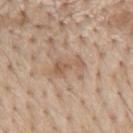<lesion>
  <biopsy_status>not biopsied; imaged during a skin examination</biopsy_status>
  <automated_metrics>
    <cielab_L>57</cielab_L>
    <cielab_a>17</cielab_a>
    <cielab_b>30</cielab_b>
    <vs_skin_darker_L>8.0</vs_skin_darker_L>
    <vs_skin_contrast_norm>5.5</vs_skin_contrast_norm>
    <border_irregularity_0_10>6.5</border_irregularity_0_10>
    <color_variation_0_10>2.0</color_variation_0_10>
    <peripheral_color_asymmetry>0.5</peripheral_color_asymmetry>
    <lesion_detection_confidence_0_100>100</lesion_detection_confidence_0_100>
  </automated_metrics>
  <lesion_size>
    <long_diameter_mm_approx>4.0</long_diameter_mm_approx>
  </lesion_size>
  <lighting>white-light</lighting>
  <site>mid back</site>
  <image>
    <source>total-body photography crop</source>
    <field_of_view_mm>15</field_of_view_mm>
  </image>
  <patient>
    <sex>male</sex>
    <age_approx>70</age_approx>
  </patient>
</lesion>